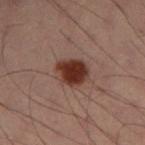Q: Is there a histopathology result?
A: imaged on a skin check; not biopsied
Q: Lesion size?
A: about 4 mm
Q: Lesion location?
A: the left lower leg
Q: What is the imaging modality?
A: ~15 mm crop, total-body skin-cancer survey
Q: What did automated image analysis measure?
A: an area of roughly 9.5 mm², an outline eccentricity of about 0.6 (0 = round, 1 = elongated), and two-axis asymmetry of about 0.15; a lesion color around L≈27 a*≈18 b*≈21 in CIELAB, roughly 12 lightness units darker than nearby skin, and a normalized border contrast of about 12.5
Q: How was the tile lit?
A: cross-polarized illumination
Q: Patient demographics?
A: male, roughly 55 years of age